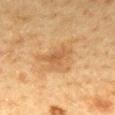Captured during whole-body skin photography for melanoma surveillance; the lesion was not biopsied. A female patient, about 50 years old. The lesion is on the back. A lesion tile, about 15 mm wide, cut from a 3D total-body photograph. The recorded lesion diameter is about 4.5 mm.